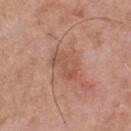Notes:
• notes · imaged on a skin check; not biopsied